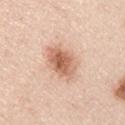follow-up: imaged on a skin check; not biopsied | size: ~5 mm (longest diameter) | automated metrics: a lesion–skin lightness drop of about 14 and a lesion-to-skin contrast of about 8.5 (normalized; higher = more distinct); a nevus-likeness score of about 90/100 and lesion-presence confidence of about 100/100 | location: the left upper arm | image: ~15 mm tile from a whole-body skin photo | patient: male, in their mid- to late 40s | lighting: white-light illumination.